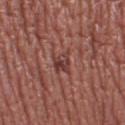Recorded during total-body skin imaging; not selected for excision or biopsy.
The total-body-photography lesion software estimated a lesion color around L≈39 a*≈25 b*≈23 in CIELAB and a normalized border contrast of about 7.5. It also reported border irregularity of about 3.5 on a 0–10 scale, a color-variation rating of about 7/10, and peripheral color asymmetry of about 2.5. The software also gave an automated nevus-likeness rating near 0 out of 100 and a detector confidence of about 75 out of 100 that the crop contains a lesion.
This is a white-light tile.
The lesion's longest dimension is about 3 mm.
The lesion is on the leg.
A female patient roughly 60 years of age.
A roughly 15 mm field-of-view crop from a total-body skin photograph.